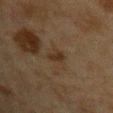This lesion was catalogued during total-body skin photography and was not selected for biopsy.
The patient is a male in their mid- to late 60s.
A region of skin cropped from a whole-body photographic capture, roughly 15 mm wide.
From the back.